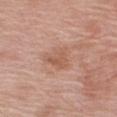body site — the right upper arm
image — ~15 mm crop, total-body skin-cancer survey
patient — male, aged 63–67